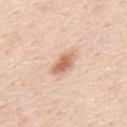Assessment:
No biopsy was performed on this lesion — it was imaged during a full skin examination and was not determined to be concerning.